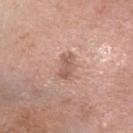biopsy_status: not biopsied; imaged during a skin examination
lesion_size:
  long_diameter_mm_approx: 3.0
lighting: white-light
site: head or neck
automated_metrics:
  area_mm2_approx: 4.5
  eccentricity: 0.85
  shape_asymmetry: 0.35
  border_irregularity_0_10: 3.5
  nevus_likeness_0_100: 0
patient:
  sex: female
  age_approx: 40
image:
  source: total-body photography crop
  field_of_view_mm: 15